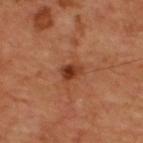{
  "biopsy_status": "not biopsied; imaged during a skin examination",
  "lesion_size": {
    "long_diameter_mm_approx": 2.5
  },
  "site": "upper back",
  "patient": {
    "sex": "male",
    "age_approx": 60
  },
  "image": {
    "source": "total-body photography crop",
    "field_of_view_mm": 15
  }
}